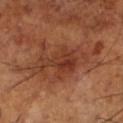This lesion was catalogued during total-body skin photography and was not selected for biopsy. Located on the leg. The lesion-visualizer software estimated a nevus-likeness score of about 10/100 and a detector confidence of about 100 out of 100 that the crop contains a lesion. A 15 mm crop from a total-body photograph taken for skin-cancer surveillance. A male subject, about 65 years old. Captured under cross-polarized illumination.